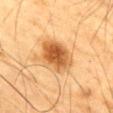This lesion was catalogued during total-body skin photography and was not selected for biopsy.
A male subject, about 85 years old or older.
The total-body-photography lesion software estimated a shape eccentricity near 0.6 and a symmetry-axis asymmetry near 0.15. The analysis additionally found a border-irregularity index near 1.5/10 and a within-lesion color-variation index near 6/10.
A 15 mm close-up tile from a total-body photography series done for melanoma screening.
The lesion is on the chest.
Approximately 4.5 mm at its widest.